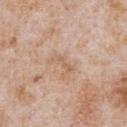No biopsy was performed on this lesion — it was imaged during a full skin examination and was not determined to be concerning. A roughly 15 mm field-of-view crop from a total-body skin photograph. Captured under white-light illumination. The subject is a male aged 63 to 67. On the chest. Automated tile analysis of the lesion measured two-axis asymmetry of about 0.4. The analysis additionally found a classifier nevus-likeness of about 0/100 and a detector confidence of about 100 out of 100 that the crop contains a lesion.